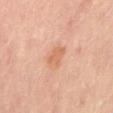Clinical impression:
The lesion was photographed on a routine skin check and not biopsied; there is no pathology result.
Context:
Automated image analysis of the tile measured a shape eccentricity near 0.75 and a symmetry-axis asymmetry near 0.3. The software also gave a mean CIELAB color near L≈62 a*≈24 b*≈35 and a lesion-to-skin contrast of about 6 (normalized; higher = more distinct). It also reported a border-irregularity rating of about 2.5/10 and peripheral color asymmetry of about 0.5. And it measured an automated nevus-likeness rating near 30 out of 100 and a lesion-detection confidence of about 100/100. The lesion's longest dimension is about 3 mm. The patient is a female aged approximately 50. Located on the front of the torso. A roughly 15 mm field-of-view crop from a total-body skin photograph. Imaged with cross-polarized lighting.